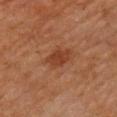image source: ~15 mm tile from a whole-body skin photo | site: the arm | patient: male, approximately 65 years of age.